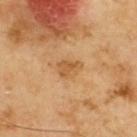Impression: The lesion was tiled from a total-body skin photograph and was not biopsied. Context: On the upper back. The recorded lesion diameter is about 3 mm. Cropped from a whole-body photographic skin survey; the tile spans about 15 mm. Captured under cross-polarized illumination. The patient is a male approximately 70 years of age.